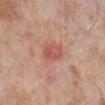Impression: This lesion was catalogued during total-body skin photography and was not selected for biopsy. Image and clinical context: About 3 mm across. A roughly 15 mm field-of-view crop from a total-body skin photograph. Captured under white-light illumination. The subject is a male aged approximately 80. From the leg. The lesion-visualizer software estimated a footprint of about 6 mm² and a shape eccentricity near 0.7. The software also gave border irregularity of about 2 on a 0–10 scale, a color-variation rating of about 3/10, and peripheral color asymmetry of about 1. The software also gave a nevus-likeness score of about 0/100.